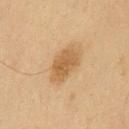follow-up: total-body-photography surveillance lesion; no biopsy | lesion size: ≈4.5 mm | TBP lesion metrics: a lesion area of about 9.5 mm² and a shape eccentricity near 0.85; an average lesion color of about L≈48 a*≈15 b*≈34 (CIELAB), about 9 CIELAB-L* units darker than the surrounding skin, and a lesion-to-skin contrast of about 7 (normalized; higher = more distinct); a border-irregularity index near 2.5/10, a within-lesion color-variation index near 2.5/10, and radial color variation of about 1; a nevus-likeness score of about 75/100 | patient: male, about 60 years old | acquisition: ~15 mm crop, total-body skin-cancer survey | anatomic site: the chest.